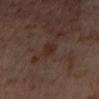Recorded during total-body skin imaging; not selected for excision or biopsy.
A female subject aged 53 to 57.
The total-body-photography lesion software estimated an average lesion color of about L≈27 a*≈17 b*≈22 (CIELAB), about 6 CIELAB-L* units darker than the surrounding skin, and a normalized lesion–skin contrast near 7. It also reported a border-irregularity rating of about 2.5/10, a within-lesion color-variation index near 1/10, and radial color variation of about 0. The software also gave a detector confidence of about 100 out of 100 that the crop contains a lesion.
On the left thigh.
A 15 mm close-up extracted from a 3D total-body photography capture.